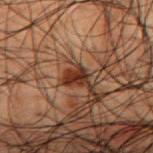– workup · catalogued during a skin exam; not biopsied
– illumination · cross-polarized
– size · ≈2.5 mm
– acquisition · total-body-photography crop, ~15 mm field of view
– patient · male, aged 48 to 52
– site · the mid back